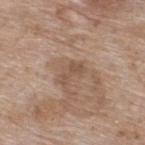| feature | finding |
|---|---|
| notes | no biopsy performed (imaged during a skin exam) |
| image-analysis metrics | an area of roughly 3.5 mm², an outline eccentricity of about 0.9 (0 = round, 1 = elongated), and two-axis asymmetry of about 0.55; an average lesion color of about L≈51 a*≈17 b*≈28 (CIELAB), about 7 CIELAB-L* units darker than the surrounding skin, and a normalized border contrast of about 5.5; border irregularity of about 6.5 on a 0–10 scale, a color-variation rating of about 0/10, and radial color variation of about 0; a classifier nevus-likeness of about 0/100 and a lesion-detection confidence of about 100/100 |
| tile lighting | white-light |
| size | ~3 mm (longest diameter) |
| anatomic site | the upper back |
| subject | female, aged 73–77 |
| image source | total-body-photography crop, ~15 mm field of view |